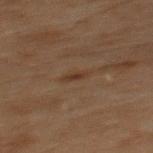notes — total-body-photography surveillance lesion; no biopsy
subject — male, in their 70s
site — the mid back
acquisition — 15 mm crop, total-body photography
size — about 2.5 mm